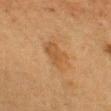notes: catalogued during a skin exam; not biopsied
imaging modality: 15 mm crop, total-body photography
patient: female, aged approximately 55
tile lighting: cross-polarized illumination
lesion diameter: about 3.5 mm
anatomic site: the head or neck
TBP lesion metrics: an eccentricity of roughly 0.75 and a shape-asymmetry score of about 0.25 (0 = symmetric); a lesion color around L≈44 a*≈18 b*≈34 in CIELAB, roughly 6 lightness units darker than nearby skin, and a normalized lesion–skin contrast near 5.5; internal color variation of about 1.5 on a 0–10 scale and peripheral color asymmetry of about 0.5; an automated nevus-likeness rating near 15 out of 100 and lesion-presence confidence of about 100/100